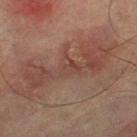Assessment:
The lesion was photographed on a routine skin check and not biopsied; there is no pathology result.
Context:
The lesion is on the left thigh. A male patient, aged 73 to 77. A lesion tile, about 15 mm wide, cut from a 3D total-body photograph.